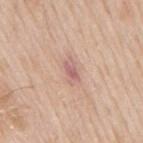Recorded during total-body skin imaging; not selected for excision or biopsy.
Captured under white-light illumination.
A male subject, roughly 65 years of age.
The lesion is on the mid back.
A 15 mm close-up extracted from a 3D total-body photography capture.
The lesion-visualizer software estimated a lesion area of about 4 mm², a shape eccentricity near 0.9, and a symmetry-axis asymmetry near 0.25. The analysis additionally found an average lesion color of about L≈62 a*≈22 b*≈24 (CIELAB) and roughly 9 lightness units darker than nearby skin. The analysis additionally found a border-irregularity index near 3/10 and a within-lesion color-variation index near 5/10.